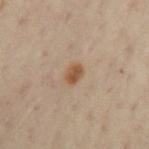notes: imaged on a skin check; not biopsied.